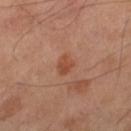Imaged during a routine full-body skin examination; the lesion was not biopsied and no histopathology is available. From the leg. The lesion's longest dimension is about 2.5 mm. The tile uses cross-polarized illumination. A male patient, aged 63 to 67. Cropped from a total-body skin-imaging series; the visible field is about 15 mm.